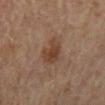Case summary:
* follow-up — total-body-photography surveillance lesion; no biopsy
* subject — male, in their mid-60s
* image source — total-body-photography crop, ~15 mm field of view
* site — the lower back
* lesion size — about 4 mm
* image-analysis metrics — an area of roughly 7 mm² and two-axis asymmetry of about 0.3; a mean CIELAB color near L≈38 a*≈18 b*≈27, roughly 8 lightness units darker than nearby skin, and a normalized border contrast of about 8; a classifier nevus-likeness of about 60/100 and lesion-presence confidence of about 100/100
* lighting — cross-polarized illumination